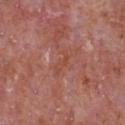Assessment: The lesion was tiled from a total-body skin photograph and was not biopsied. Image and clinical context: A close-up tile cropped from a whole-body skin photograph, about 15 mm across. Longest diameter approximately 3 mm. Captured under white-light illumination. An algorithmic analysis of the crop reported a lesion color around L≈48 a*≈27 b*≈30 in CIELAB, a lesion–skin lightness drop of about 5, and a normalized border contrast of about 5. It also reported a border-irregularity rating of about 7/10 and peripheral color asymmetry of about 0. The subject is a male in their mid- to late 60s. The lesion is located on the chest.